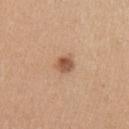Captured during whole-body skin photography for melanoma surveillance; the lesion was not biopsied.
On the left upper arm.
A female subject about 30 years old.
Longest diameter approximately 2.5 mm.
Automated tile analysis of the lesion measured a border-irregularity rating of about 2/10 and peripheral color asymmetry of about 1.
A lesion tile, about 15 mm wide, cut from a 3D total-body photograph.